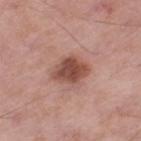<lesion>
<lesion_size>
  <long_diameter_mm_approx>4.0</long_diameter_mm_approx>
</lesion_size>
<automated_metrics>
  <vs_skin_darker_L>13.0</vs_skin_darker_L>
  <vs_skin_contrast_norm>9.5</vs_skin_contrast_norm>
  <border_irregularity_0_10>1.5</border_irregularity_0_10>
  <color_variation_0_10>3.5</color_variation_0_10>
  <peripheral_color_asymmetry>1.0</peripheral_color_asymmetry>
  <nevus_likeness_0_100>65</nevus_likeness_0_100>
</automated_metrics>
<image>
  <source>total-body photography crop</source>
  <field_of_view_mm>15</field_of_view_mm>
</image>
<lighting>white-light</lighting>
<site>left thigh</site>
<patient>
  <sex>male</sex>
  <age_approx>55</age_approx>
</patient>
</lesion>